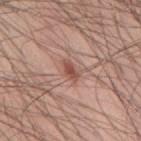Imaged during a routine full-body skin examination; the lesion was not biopsied and no histopathology is available. A male patient, about 60 years old. Measured at roughly 2.5 mm in maximum diameter. The lesion is on the mid back. An algorithmic analysis of the crop reported a lesion area of about 3 mm². It also reported a mean CIELAB color near L≈51 a*≈23 b*≈26, about 11 CIELAB-L* units darker than the surrounding skin, and a normalized lesion–skin contrast near 8. And it measured a border-irregularity index near 2/10. This is a white-light tile. A 15 mm crop from a total-body photograph taken for skin-cancer surveillance.